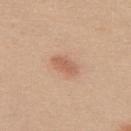Captured during whole-body skin photography for melanoma surveillance; the lesion was not biopsied. The patient is a female aged 18–22. This image is a 15 mm lesion crop taken from a total-body photograph. The lesion is located on the upper back.